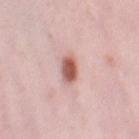<record>
  <lighting>white-light</lighting>
  <site>right thigh</site>
  <patient>
    <sex>female</sex>
    <age_approx>40</age_approx>
  </patient>
  <image>
    <source>total-body photography crop</source>
    <field_of_view_mm>15</field_of_view_mm>
  </image>
  <automated_metrics>
    <area_mm2_approx>5.0</area_mm2_approx>
    <eccentricity>0.65</eccentricity>
    <shape_asymmetry>0.15</shape_asymmetry>
    <cielab_L>58</cielab_L>
    <cielab_a>25</cielab_a>
    <cielab_b>25</cielab_b>
    <vs_skin_contrast_norm>10.5</vs_skin_contrast_norm>
    <border_irregularity_0_10>1.0</border_irregularity_0_10>
    <peripheral_color_asymmetry>1.5</peripheral_color_asymmetry>
  </automated_metrics>
</record>